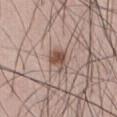Clinical impression: This lesion was catalogued during total-body skin photography and was not selected for biopsy. Image and clinical context: A 15 mm crop from a total-body photograph taken for skin-cancer surveillance. From the abdomen. The subject is a male aged approximately 60. Approximately 2.5 mm at its widest. Imaged with white-light lighting.